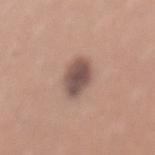| key | value |
|---|---|
| biopsy status | total-body-photography surveillance lesion; no biopsy |
| lighting | white-light |
| image | ~15 mm tile from a whole-body skin photo |
| patient | male, aged approximately 30 |
| lesion size | about 4.5 mm |
| site | the mid back |